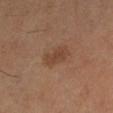The lesion was tiled from a total-body skin photograph and was not biopsied. Captured under cross-polarized illumination. About 3 mm across. A close-up tile cropped from a whole-body skin photograph, about 15 mm across. The lesion-visualizer software estimated a footprint of about 4.5 mm², an eccentricity of roughly 0.85, and a shape-asymmetry score of about 0.3 (0 = symmetric). And it measured an average lesion color of about L≈41 a*≈19 b*≈30 (CIELAB), roughly 7 lightness units darker than nearby skin, and a normalized border contrast of about 6.5. It also reported border irregularity of about 3 on a 0–10 scale, internal color variation of about 1 on a 0–10 scale, and a peripheral color-asymmetry measure near 0.5. And it measured a nevus-likeness score of about 15/100 and a lesion-detection confidence of about 100/100. The patient is a female aged 48 to 52.